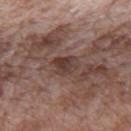follow-up: catalogued during a skin exam; not biopsied | subject: male, approximately 70 years of age | automated lesion analysis: a normalized lesion–skin contrast near 7.5; an automated nevus-likeness rating near 0 out of 100 and a lesion-detection confidence of about 95/100 | size: ~3.5 mm (longest diameter) | imaging modality: 15 mm crop, total-body photography | tile lighting: white-light | location: the back.